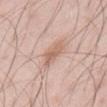workup: catalogued during a skin exam; not biopsied
subject: male, in their mid-40s
anatomic site: the abdomen
image source: ~15 mm crop, total-body skin-cancer survey
lighting: white-light illumination
automated lesion analysis: an area of roughly 7 mm², a shape eccentricity near 0.9, and a symmetry-axis asymmetry near 0.3; border irregularity of about 4 on a 0–10 scale, a within-lesion color-variation index near 3.5/10, and peripheral color asymmetry of about 1.5; a nevus-likeness score of about 0/100 and a detector confidence of about 100 out of 100 that the crop contains a lesion
diameter: about 4.5 mm